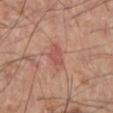On the left lower leg. This image is a 15 mm lesion crop taken from a total-body photograph. The patient is a male in their 60s. This is a cross-polarized tile. Measured at roughly 3.5 mm in maximum diameter.